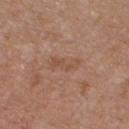Imaged during a routine full-body skin examination; the lesion was not biopsied and no histopathology is available. On the chest. A male patient aged 53 to 57. A close-up tile cropped from a whole-body skin photograph, about 15 mm across.